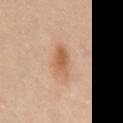Clinical impression: Recorded during total-body skin imaging; not selected for excision or biopsy. Acquisition and patient details: On the mid back. Cropped from a total-body skin-imaging series; the visible field is about 15 mm. The patient is a female about 50 years old. An algorithmic analysis of the crop reported a lesion area of about 7 mm². The analysis additionally found a border-irregularity rating of about 3/10, a color-variation rating of about 3/10, and a peripheral color-asymmetry measure near 1. It also reported a classifier nevus-likeness of about 90/100 and a lesion-detection confidence of about 100/100.